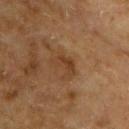Q: Was this lesion biopsied?
A: catalogued during a skin exam; not biopsied
Q: How large is the lesion?
A: ~3.5 mm (longest diameter)
Q: How was this image acquired?
A: total-body-photography crop, ~15 mm field of view
Q: What did automated image analysis measure?
A: a shape eccentricity near 0.8 and a shape-asymmetry score of about 0.4 (0 = symmetric); a border-irregularity index near 4.5/10, a color-variation rating of about 2/10, and radial color variation of about 0.5; a classifier nevus-likeness of about 0/100 and a detector confidence of about 100 out of 100 that the crop contains a lesion
Q: How was the tile lit?
A: cross-polarized
Q: What are the patient's age and sex?
A: male, in their mid-60s
Q: Where on the body is the lesion?
A: the upper back